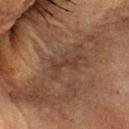{
  "biopsy_status": "not biopsied; imaged during a skin examination",
  "image": {
    "source": "total-body photography crop",
    "field_of_view_mm": 15
  },
  "lighting": "cross-polarized",
  "lesion_size": {
    "long_diameter_mm_approx": 4.0
  },
  "site": "head or neck",
  "patient": {
    "sex": "female",
    "age_approx": 60
  }
}